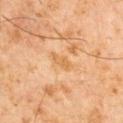| feature | finding |
|---|---|
| notes | no biopsy performed (imaged during a skin exam) |
| subject | male, approximately 60 years of age |
| acquisition | total-body-photography crop, ~15 mm field of view |
| location | the left upper arm |